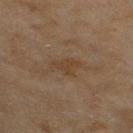Recorded during total-body skin imaging; not selected for excision or biopsy. An algorithmic analysis of the crop reported a lesion color around L≈37 a*≈14 b*≈27 in CIELAB and a lesion–skin lightness drop of about 5. The software also gave a detector confidence of about 100 out of 100 that the crop contains a lesion. Measured at roughly 3 mm in maximum diameter. A close-up tile cropped from a whole-body skin photograph, about 15 mm across. A female subject aged 58 to 62. From the right thigh.